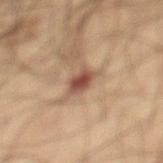Clinical impression:
Part of a total-body skin-imaging series; this lesion was reviewed on a skin check and was not flagged for biopsy.
Image and clinical context:
A 15 mm crop from a total-body photograph taken for skin-cancer surveillance. Located on the left lower leg. The subject is a male approximately 45 years of age.